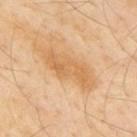follow-up = imaged on a skin check; not biopsied
lesion diameter = ≈6.5 mm
tile lighting = cross-polarized illumination
image source = ~15 mm crop, total-body skin-cancer survey
patient = male, in their mid-50s
site = the back